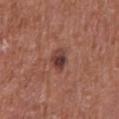The lesion was tiled from a total-body skin photograph and was not biopsied. A close-up tile cropped from a whole-body skin photograph, about 15 mm across. Located on the chest. A male subject roughly 65 years of age.